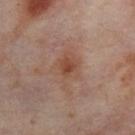follow-up: total-body-photography surveillance lesion; no biopsy
site: the right thigh
image-analysis metrics: a footprint of about 8.5 mm², an eccentricity of roughly 0.75, and two-axis asymmetry of about 0.35
acquisition: ~15 mm crop, total-body skin-cancer survey
diameter: ~5 mm (longest diameter)
patient: female, aged around 55
lighting: cross-polarized illumination